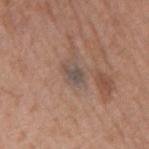The lesion was tiled from a total-body skin photograph and was not biopsied. From the mid back. The lesion's longest dimension is about 2.5 mm. Imaged with white-light lighting. Automated image analysis of the tile measured a footprint of about 3 mm², an eccentricity of roughly 0.8, and a symmetry-axis asymmetry near 0.35. It also reported a border-irregularity rating of about 3.5/10, a within-lesion color-variation index near 1/10, and radial color variation of about 0.5. And it measured a detector confidence of about 95 out of 100 that the crop contains a lesion. A region of skin cropped from a whole-body photographic capture, roughly 15 mm wide. A male subject aged 73 to 77.